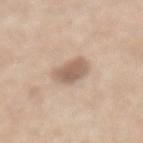workup: imaged on a skin check; not biopsied
imaging modality: total-body-photography crop, ~15 mm field of view
patient: female, in their 60s
location: the mid back